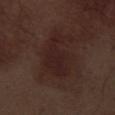{
  "biopsy_status": "not biopsied; imaged during a skin examination",
  "lesion_size": {
    "long_diameter_mm_approx": 6.0
  },
  "lighting": "white-light",
  "automated_metrics": {
    "area_mm2_approx": 16.0,
    "eccentricity": 0.8,
    "shape_asymmetry": 0.35,
    "vs_skin_darker_L": 5.0,
    "vs_skin_contrast_norm": 6.5
  },
  "patient": {
    "sex": "male",
    "age_approx": 70
  },
  "image": {
    "source": "total-body photography crop",
    "field_of_view_mm": 15
  },
  "site": "leg"
}